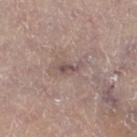{"biopsy_status": "not biopsied; imaged during a skin examination", "image": {"source": "total-body photography crop", "field_of_view_mm": 15}, "site": "right thigh", "lighting": "white-light", "lesion_size": {"long_diameter_mm_approx": 3.0}, "patient": {"sex": "male", "age_approx": 80}}